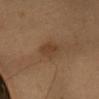{
  "biopsy_status": "not biopsied; imaged during a skin examination",
  "image": {
    "source": "total-body photography crop",
    "field_of_view_mm": 15
  },
  "automated_metrics": {
    "eccentricity": 0.65,
    "shape_asymmetry": 0.2,
    "cielab_L": 38,
    "cielab_a": 17,
    "cielab_b": 30,
    "vs_skin_contrast_norm": 6.0,
    "border_irregularity_0_10": 2.5,
    "peripheral_color_asymmetry": 0.5,
    "lesion_detection_confidence_0_100": 100
  },
  "lighting": "cross-polarized",
  "site": "head or neck",
  "lesion_size": {
    "long_diameter_mm_approx": 2.5
  },
  "patient": {
    "sex": "female",
    "age_approx": 25
  }
}